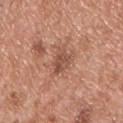Part of a total-body skin-imaging series; this lesion was reviewed on a skin check and was not flagged for biopsy. From the upper back. Captured under white-light illumination. This image is a 15 mm lesion crop taken from a total-body photograph. Automated image analysis of the tile measured a footprint of about 5.5 mm², an eccentricity of roughly 0.65, and two-axis asymmetry of about 0.35. And it measured a lesion color around L≈51 a*≈23 b*≈29 in CIELAB, a lesion–skin lightness drop of about 9, and a lesion-to-skin contrast of about 6.5 (normalized; higher = more distinct). The software also gave a within-lesion color-variation index near 3.5/10 and radial color variation of about 1.5. Longest diameter approximately 3 mm. A male patient, approximately 55 years of age.